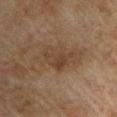workup: no biopsy performed (imaged during a skin exam); diameter: about 4.5 mm; image source: total-body-photography crop, ~15 mm field of view; illumination: cross-polarized illumination; site: the left upper arm; patient: male, about 75 years old.